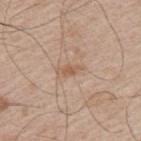Imaged during a routine full-body skin examination; the lesion was not biopsied and no histopathology is available.
A male subject aged 63 to 67.
On the left upper arm.
An algorithmic analysis of the crop reported a lesion area of about 3 mm² and a symmetry-axis asymmetry near 0.35.
Measured at roughly 3 mm in maximum diameter.
Cropped from a whole-body photographic skin survey; the tile spans about 15 mm.